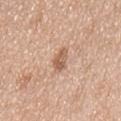lesion size — ~3.5 mm (longest diameter); anatomic site — the chest; patient — male, roughly 65 years of age; imaging modality — total-body-photography crop, ~15 mm field of view; lighting — white-light illumination.